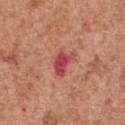Q: Was this lesion biopsied?
A: total-body-photography surveillance lesion; no biopsy
Q: Lesion location?
A: the chest
Q: Patient demographics?
A: male, in their mid- to late 60s
Q: What did automated image analysis measure?
A: an automated nevus-likeness rating near 0 out of 100 and a detector confidence of about 100 out of 100 that the crop contains a lesion
Q: How large is the lesion?
A: about 3 mm
Q: Illumination type?
A: white-light
Q: What is the imaging modality?
A: total-body-photography crop, ~15 mm field of view